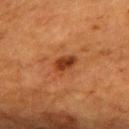| field | value |
|---|---|
| biopsy status | total-body-photography surveillance lesion; no biopsy |
| image-analysis metrics | an eccentricity of roughly 0.75 and a symmetry-axis asymmetry near 0.3; a mean CIELAB color near L≈33 a*≈24 b*≈33, roughly 10 lightness units darker than nearby skin, and a normalized lesion–skin contrast near 9; a border-irregularity index near 2.5/10, a color-variation rating of about 3.5/10, and a peripheral color-asymmetry measure near 1; a classifier nevus-likeness of about 85/100 and a lesion-detection confidence of about 100/100 |
| lighting | cross-polarized illumination |
| image source | ~15 mm tile from a whole-body skin photo |
| body site | the upper back |
| patient | female, in their mid- to late 50s |